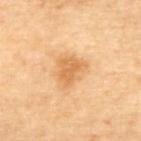Clinical impression: This lesion was catalogued during total-body skin photography and was not selected for biopsy. Clinical summary: From the upper back. A female subject roughly 65 years of age. A lesion tile, about 15 mm wide, cut from a 3D total-body photograph.